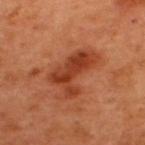Assessment:
Imaged during a routine full-body skin examination; the lesion was not biopsied and no histopathology is available.
Clinical summary:
The lesion is located on the upper back. A region of skin cropped from a whole-body photographic capture, roughly 15 mm wide. A male subject, about 50 years old. Captured under cross-polarized illumination. The total-body-photography lesion software estimated a mean CIELAB color near L≈42 a*≈31 b*≈37, about 11 CIELAB-L* units darker than the surrounding skin, and a normalized border contrast of about 8.5. The software also gave border irregularity of about 5 on a 0–10 scale. Approximately 6.5 mm at its widest.